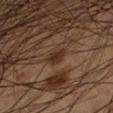Background: Measured at roughly 3 mm in maximum diameter. A 15 mm crop from a total-body photograph taken for skin-cancer surveillance. Automated image analysis of the tile measured an area of roughly 3.5 mm² and a symmetry-axis asymmetry near 0.35. And it measured a mean CIELAB color near L≈28 a*≈17 b*≈25 and a lesion–skin lightness drop of about 8. The software also gave a border-irregularity rating of about 3.5/10 and a color-variation rating of about 1.5/10. Located on the left lower leg. Captured under cross-polarized illumination. A male subject, in their mid- to late 40s.